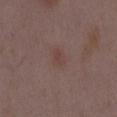Q: Is there a histopathology result?
A: total-body-photography surveillance lesion; no biopsy
Q: Lesion size?
A: ~2.5 mm (longest diameter)
Q: Patient demographics?
A: female, about 35 years old
Q: How was the tile lit?
A: white-light
Q: Lesion location?
A: the back
Q: How was this image acquired?
A: total-body-photography crop, ~15 mm field of view
Q: Automated lesion metrics?
A: a shape eccentricity near 0.75 and two-axis asymmetry of about 0.25